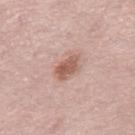The lesion was tiled from a total-body skin photograph and was not biopsied.
A female patient, aged around 65.
Cropped from a whole-body photographic skin survey; the tile spans about 15 mm.
The recorded lesion diameter is about 3.5 mm.
On the leg.